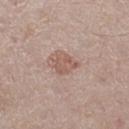This lesion was catalogued during total-body skin photography and was not selected for biopsy.
Captured under white-light illumination.
A region of skin cropped from a whole-body photographic capture, roughly 15 mm wide.
From the left thigh.
About 2.5 mm across.
A male patient approximately 65 years of age.